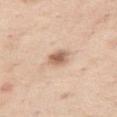About 2.5 mm across.
A female patient, aged 53–57.
A close-up tile cropped from a whole-body skin photograph, about 15 mm across.
The lesion is located on the right thigh.